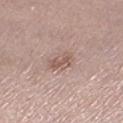Notes:
- workup · no biopsy performed (imaged during a skin exam)
- anatomic site · the right thigh
- imaging modality · 15 mm crop, total-body photography
- patient · female, roughly 65 years of age